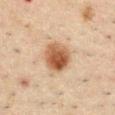Notes:
- notes · imaged on a skin check; not biopsied
- image · ~15 mm crop, total-body skin-cancer survey
- automated metrics · a lesion area of about 11 mm², an eccentricity of roughly 0.4, and two-axis asymmetry of about 0.15; a border-irregularity rating of about 1.5/10, a color-variation rating of about 6/10, and a peripheral color-asymmetry measure near 2; an automated nevus-likeness rating near 100 out of 100 and a lesion-detection confidence of about 100/100
- lesion diameter · about 3.5 mm
- tile lighting · cross-polarized
- subject · male, in their 50s
- location · the abdomen